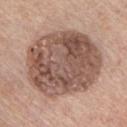site: chest
patient:
  sex: male
  age_approx: 60
image:
  source: total-body photography crop
  field_of_view_mm: 15
automated_metrics:
  area_mm2_approx: 60.0
  shape_asymmetry: 0.1
  border_irregularity_0_10: 1.5
  color_variation_0_10: 7.0
  peripheral_color_asymmetry: 2.5
  nevus_likeness_0_100: 25
  lesion_detection_confidence_0_100: 100
lesion_size:
  long_diameter_mm_approx: 9.0